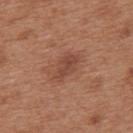| field | value |
|---|---|
| workup | no biopsy performed (imaged during a skin exam) |
| location | the upper back |
| image source | total-body-photography crop, ~15 mm field of view |
| TBP lesion metrics | roughly 8 lightness units darker than nearby skin and a normalized lesion–skin contrast near 6; lesion-presence confidence of about 100/100 |
| lighting | white-light illumination |
| patient | female, aged around 40 |
| diameter | ~3.5 mm (longest diameter) |